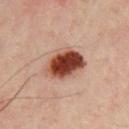Captured during whole-body skin photography for melanoma surveillance; the lesion was not biopsied.
The subject is a male approximately 45 years of age.
A roughly 15 mm field-of-view crop from a total-body skin photograph.
From the chest.